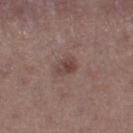Part of a total-body skin-imaging series; this lesion was reviewed on a skin check and was not flagged for biopsy. The tile uses white-light illumination. A 15 mm close-up extracted from a 3D total-body photography capture. A female patient approximately 20 years of age. Measured at roughly 3 mm in maximum diameter. The lesion is on the left thigh.